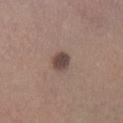follow-up: imaged on a skin check; not biopsied
acquisition: ~15 mm tile from a whole-body skin photo
patient: male, about 45 years old
anatomic site: the left lower leg